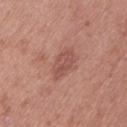Assessment: This lesion was catalogued during total-body skin photography and was not selected for biopsy. Context: The patient is a female about 40 years old. A region of skin cropped from a whole-body photographic capture, roughly 15 mm wide. Longest diameter approximately 3.5 mm. Imaged with white-light lighting. On the left thigh.